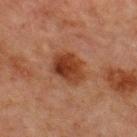workup: total-body-photography surveillance lesion; no biopsy | lesion size: ≈4.5 mm | patient: male, roughly 65 years of age | anatomic site: the chest | imaging modality: 15 mm crop, total-body photography | illumination: cross-polarized.